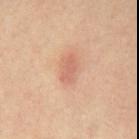notes = imaged on a skin check; not biopsied | TBP lesion metrics = an area of roughly 5 mm², a shape eccentricity near 0.85, and two-axis asymmetry of about 0.25; a classifier nevus-likeness of about 25/100 and a lesion-detection confidence of about 100/100 | image = total-body-photography crop, ~15 mm field of view | patient = male, in their mid- to late 50s | anatomic site = the front of the torso.